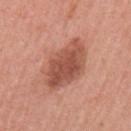Captured during whole-body skin photography for melanoma surveillance; the lesion was not biopsied.
From the left upper arm.
The lesion's longest dimension is about 6 mm.
A female patient aged approximately 55.
Imaged with white-light lighting.
A 15 mm close-up extracted from a 3D total-body photography capture.